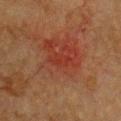notes=total-body-photography surveillance lesion; no biopsy
patient=female, roughly 55 years of age
image source=~15 mm tile from a whole-body skin photo
image-analysis metrics=an average lesion color of about L≈30 a*≈28 b*≈28 (CIELAB) and a normalized border contrast of about 5; a border-irregularity index near 2.5/10, internal color variation of about 2 on a 0–10 scale, and peripheral color asymmetry of about 0.5
site=the chest
lesion diameter=about 3 mm